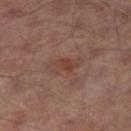{"biopsy_status": "not biopsied; imaged during a skin examination", "lighting": "cross-polarized", "lesion_size": {"long_diameter_mm_approx": 4.0}, "site": "leg", "patient": {"sex": "male", "age_approx": 65}, "image": {"source": "total-body photography crop", "field_of_view_mm": 15}}